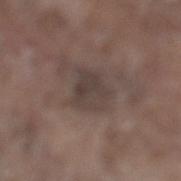Assessment: No biopsy was performed on this lesion — it was imaged during a full skin examination and was not determined to be concerning. Clinical summary: Automated tile analysis of the lesion measured a lesion area of about 9.5 mm² and a symmetry-axis asymmetry near 0.3. And it measured an average lesion color of about L≈39 a*≈12 b*≈18 (CIELAB), a lesion–skin lightness drop of about 7, and a normalized lesion–skin contrast near 6. And it measured a classifier nevus-likeness of about 0/100 and lesion-presence confidence of about 90/100. The patient is a male aged 78–82. Cropped from a whole-body photographic skin survey; the tile spans about 15 mm. Located on the leg. The recorded lesion diameter is about 3.5 mm.